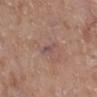workup: no biopsy performed (imaged during a skin exam)
lesion size: ≈3 mm
imaging modality: total-body-photography crop, ~15 mm field of view
site: the right lower leg
lighting: white-light illumination
subject: female, aged approximately 75
image-analysis metrics: an average lesion color of about L≈50 a*≈18 b*≈19 (CIELAB) and a normalized border contrast of about 7; a border-irregularity rating of about 4/10, internal color variation of about 0 on a 0–10 scale, and radial color variation of about 0; a classifier nevus-likeness of about 0/100 and a lesion-detection confidence of about 65/100